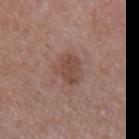The lesion was photographed on a routine skin check and not biopsied; there is no pathology result. The tile uses white-light illumination. On the arm. A region of skin cropped from a whole-body photographic capture, roughly 15 mm wide. A female subject, approximately 40 years of age.